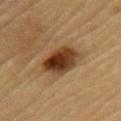Impression: Part of a total-body skin-imaging series; this lesion was reviewed on a skin check and was not flagged for biopsy. Acquisition and patient details: The total-body-photography lesion software estimated an area of roughly 12 mm², an eccentricity of roughly 0.45, and a shape-asymmetry score of about 0.15 (0 = symmetric). The analysis additionally found border irregularity of about 1.5 on a 0–10 scale, internal color variation of about 7 on a 0–10 scale, and radial color variation of about 2. The analysis additionally found a classifier nevus-likeness of about 100/100. A lesion tile, about 15 mm wide, cut from a 3D total-body photograph. Approximately 4 mm at its widest. A male subject, in their mid- to late 80s or older. From the left upper arm.